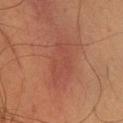Q: Was a biopsy performed?
A: imaged on a skin check; not biopsied
Q: Automated lesion metrics?
A: a border-irregularity rating of about 4/10 and a peripheral color-asymmetry measure near 0.5
Q: Where on the body is the lesion?
A: the chest
Q: Who is the patient?
A: male, aged around 35
Q: How was this image acquired?
A: ~15 mm tile from a whole-body skin photo
Q: Illumination type?
A: cross-polarized illumination